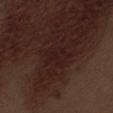{
  "patient": {
    "sex": "male",
    "age_approx": 70
  },
  "site": "abdomen",
  "lighting": "white-light",
  "automated_metrics": {
    "eccentricity": 0.65,
    "shape_asymmetry": 0.35,
    "border_irregularity_0_10": 4.5,
    "color_variation_0_10": 1.5,
    "peripheral_color_asymmetry": 0.5,
    "nevus_likeness_0_100": 0,
    "lesion_detection_confidence_0_100": 95
  },
  "image": {
    "source": "total-body photography crop",
    "field_of_view_mm": 15
  },
  "lesion_size": {
    "long_diameter_mm_approx": 3.0
  }
}